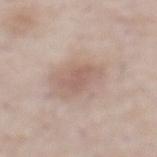follow-up = imaged on a skin check; not biopsied | site = the abdomen | automated metrics = a lesion area of about 6 mm², a shape eccentricity near 0.75, and two-axis asymmetry of about 0.25; an average lesion color of about L≈59 a*≈17 b*≈24 (CIELAB), about 7 CIELAB-L* units darker than the surrounding skin, and a normalized lesion–skin contrast near 5; an automated nevus-likeness rating near 10 out of 100 | tile lighting = white-light | image = ~15 mm tile from a whole-body skin photo | diameter = ~3 mm (longest diameter) | subject = male, aged 53 to 57.